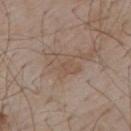- follow-up: total-body-photography surveillance lesion; no biopsy
- anatomic site: the upper back
- illumination: white-light
- patient: male, aged approximately 55
- automated lesion analysis: a lesion color around L≈52 a*≈15 b*≈26 in CIELAB and a normalized lesion–skin contrast near 4.5; a border-irregularity index near 3/10, a color-variation rating of about 2.5/10, and radial color variation of about 1; an automated nevus-likeness rating near 0 out of 100 and a lesion-detection confidence of about 100/100
- acquisition: ~15 mm tile from a whole-body skin photo
- lesion size: about 3.5 mm